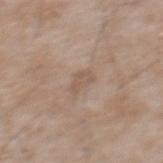Findings:
• notes: total-body-photography surveillance lesion; no biopsy
• TBP lesion metrics: a footprint of about 3.5 mm², an eccentricity of roughly 0.75, and two-axis asymmetry of about 0.3; border irregularity of about 3 on a 0–10 scale, a within-lesion color-variation index near 2.5/10, and peripheral color asymmetry of about 1; a classifier nevus-likeness of about 0/100
• body site: the mid back
• patient: male, aged 48–52
• image: total-body-photography crop, ~15 mm field of view
• lesion size: ~2.5 mm (longest diameter)